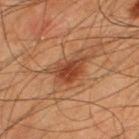Captured during whole-body skin photography for melanoma surveillance; the lesion was not biopsied. From the back. The tile uses cross-polarized illumination. Cropped from a total-body skin-imaging series; the visible field is about 15 mm. A male subject, in their mid- to late 40s. The recorded lesion diameter is about 5 mm.